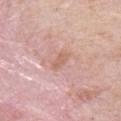imaging modality: ~15 mm tile from a whole-body skin photo
subject: male, in their 40s
site: the chest
automated lesion analysis: a lesion color around L≈62 a*≈23 b*≈27 in CIELAB and about 7 CIELAB-L* units darker than the surrounding skin; border irregularity of about 2 on a 0–10 scale, internal color variation of about 1 on a 0–10 scale, and peripheral color asymmetry of about 0; a nevus-likeness score of about 0/100 and a detector confidence of about 100 out of 100 that the crop contains a lesion